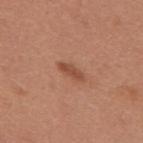Q: Was a biopsy performed?
A: imaged on a skin check; not biopsied
Q: What is the anatomic site?
A: the upper back
Q: Lesion size?
A: about 3.5 mm
Q: What lighting was used for the tile?
A: white-light
Q: What are the patient's age and sex?
A: female, aged 48–52
Q: What is the imaging modality?
A: ~15 mm tile from a whole-body skin photo